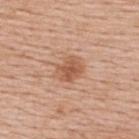This lesion was catalogued during total-body skin photography and was not selected for biopsy. This is a white-light tile. A female subject aged 63 to 67. The recorded lesion diameter is about 3.5 mm. On the upper back. A roughly 15 mm field-of-view crop from a total-body skin photograph.